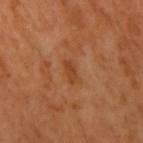The lesion was tiled from a total-body skin photograph and was not biopsied.
Approximately 2.5 mm at its widest.
The lesion is on the right upper arm.
A close-up tile cropped from a whole-body skin photograph, about 15 mm across.
A female subject, aged around 55.
This is a cross-polarized tile.